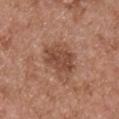Clinical impression: Recorded during total-body skin imaging; not selected for excision or biopsy. Context: The lesion's longest dimension is about 4 mm. The total-body-photography lesion software estimated an outline eccentricity of about 0.6 (0 = round, 1 = elongated) and a symmetry-axis asymmetry near 0.2. The analysis additionally found a border-irregularity rating of about 3/10, internal color variation of about 2.5 on a 0–10 scale, and a peripheral color-asymmetry measure near 1. A lesion tile, about 15 mm wide, cut from a 3D total-body photograph. This is a white-light tile. A male subject about 55 years old. On the front of the torso.